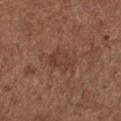workup: no biopsy performed (imaged during a skin exam)
site: the left lower leg
size: about 3.5 mm
subject: female, approximately 55 years of age
imaging modality: ~15 mm crop, total-body skin-cancer survey
TBP lesion metrics: a normalized lesion–skin contrast near 5.5; a border-irregularity rating of about 3/10, a within-lesion color-variation index near 3/10, and a peripheral color-asymmetry measure near 1
tile lighting: white-light illumination